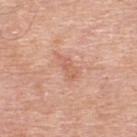follow-up: imaged on a skin check; not biopsied
subject: male, aged 58 to 62
tile lighting: white-light
automated metrics: an area of roughly 3.5 mm² and two-axis asymmetry of about 0.4; an average lesion color of about L≈61 a*≈25 b*≈32 (CIELAB), about 8 CIELAB-L* units darker than the surrounding skin, and a lesion-to-skin contrast of about 5.5 (normalized; higher = more distinct); a within-lesion color-variation index near 1.5/10
anatomic site: the upper back
imaging modality: 15 mm crop, total-body photography